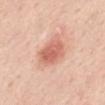No biopsy was performed on this lesion — it was imaged during a full skin examination and was not determined to be concerning.
A region of skin cropped from a whole-body photographic capture, roughly 15 mm wide.
An algorithmic analysis of the crop reported a footprint of about 9.5 mm², a shape eccentricity near 0.7, and two-axis asymmetry of about 0.15. And it measured a lesion color around L≈63 a*≈27 b*≈31 in CIELAB and a normalized border contrast of about 7.5. The software also gave a classifier nevus-likeness of about 100/100 and a lesion-detection confidence of about 100/100.
Located on the mid back.
A female subject about 45 years old.
Captured under white-light illumination.